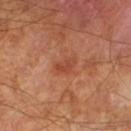Automated image analysis of the tile measured a lesion color around L≈44 a*≈29 b*≈33 in CIELAB and a normalized border contrast of about 6. A male patient aged approximately 65. A roughly 15 mm field-of-view crop from a total-body skin photograph. The recorded lesion diameter is about 2.5 mm.